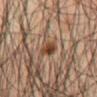No biopsy was performed on this lesion — it was imaged during a full skin examination and was not determined to be concerning. Cropped from a whole-body photographic skin survey; the tile spans about 15 mm. This is a cross-polarized tile. An algorithmic analysis of the crop reported a mean CIELAB color near L≈41 a*≈19 b*≈29, roughly 10 lightness units darker than nearby skin, and a normalized border contrast of about 9. It also reported a nevus-likeness score of about 90/100 and a detector confidence of about 100 out of 100 that the crop contains a lesion. A male subject, aged around 45. Approximately 2.5 mm at its widest. The lesion is on the front of the torso.